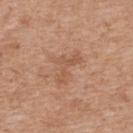Findings:
- follow-up · total-body-photography surveillance lesion; no biopsy
- image source · ~15 mm tile from a whole-body skin photo
- lesion size · about 4.5 mm
- site · the upper back
- subject · female, aged approximately 40
- automated lesion analysis · a footprint of about 7.5 mm² and an outline eccentricity of about 0.8 (0 = round, 1 = elongated); an average lesion color of about L≈55 a*≈21 b*≈32 (CIELAB) and a lesion-to-skin contrast of about 5 (normalized; higher = more distinct); a within-lesion color-variation index near 1.5/10; a nevus-likeness score of about 0/100